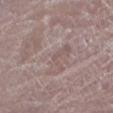Acquisition and patient details:
A female subject in their mid-60s. The lesion is located on the left lower leg. A 15 mm close-up extracted from a 3D total-body photography capture.